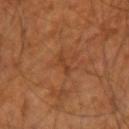{
  "biopsy_status": "not biopsied; imaged during a skin examination",
  "automated_metrics": {
    "vs_skin_darker_L": 6.0,
    "vs_skin_contrast_norm": 5.5,
    "border_irregularity_0_10": 4.5,
    "peripheral_color_asymmetry": 0.0,
    "nevus_likeness_0_100": 0,
    "lesion_detection_confidence_0_100": 95
  },
  "patient": {
    "sex": "male",
    "age_approx": 60
  },
  "lighting": "cross-polarized",
  "lesion_size": {
    "long_diameter_mm_approx": 2.5
  },
  "site": "right upper arm",
  "image": {
    "source": "total-body photography crop",
    "field_of_view_mm": 15
  }
}